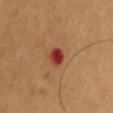Clinical impression: Recorded during total-body skin imaging; not selected for excision or biopsy. Context: This image is a 15 mm lesion crop taken from a total-body photograph. From the mid back. The tile uses cross-polarized illumination. The subject is a male roughly 55 years of age. The recorded lesion diameter is about 2.5 mm. An algorithmic analysis of the crop reported a color-variation rating of about 5/10 and radial color variation of about 1.5. It also reported an automated nevus-likeness rating near 0 out of 100 and lesion-presence confidence of about 100/100.